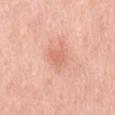  biopsy_status: not biopsied; imaged during a skin examination
  lesion_size:
    long_diameter_mm_approx: 2.5
  image:
    source: total-body photography crop
    field_of_view_mm: 15
  site: mid back
  patient:
    sex: male
    age_approx: 60